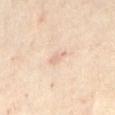biopsy status: no biopsy performed (imaged during a skin exam)
site: the abdomen
diameter: ~2.5 mm (longest diameter)
acquisition: ~15 mm tile from a whole-body skin photo
subject: female, aged 58–62